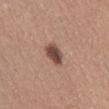notes — no biopsy performed (imaged during a skin exam); size — about 3 mm; image source — ~15 mm tile from a whole-body skin photo; subject — female, roughly 50 years of age; anatomic site — the abdomen.